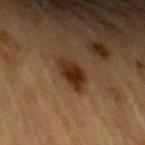follow-up: imaged on a skin check; not biopsied
subject: male, in their mid- to late 80s or older
site: the left upper arm
image source: 15 mm crop, total-body photography